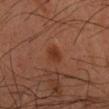This lesion was catalogued during total-body skin photography and was not selected for biopsy.
A 15 mm close-up extracted from a 3D total-body photography capture.
The lesion is on the left forearm.
A female subject aged 53–57.
Imaged with cross-polarized lighting.
Approximately 2 mm at its widest.